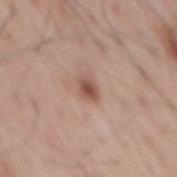Captured during whole-body skin photography for melanoma surveillance; the lesion was not biopsied. The lesion is located on the mid back. A male patient roughly 70 years of age. Cropped from a whole-body photographic skin survey; the tile spans about 15 mm.